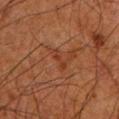Clinical summary:
The recorded lesion diameter is about 3 mm. This is a cross-polarized tile. Cropped from a whole-body photographic skin survey; the tile spans about 15 mm. On the left lower leg. A male subject aged approximately 80. The lesion-visualizer software estimated an average lesion color of about L≈29 a*≈21 b*≈27 (CIELAB), roughly 5 lightness units darker than nearby skin, and a normalized border contrast of about 5. The analysis additionally found a nevus-likeness score of about 0/100 and a detector confidence of about 95 out of 100 that the crop contains a lesion.